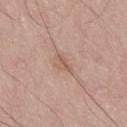Recorded during total-body skin imaging; not selected for excision or biopsy.